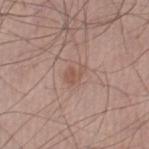{
  "image": {
    "source": "total-body photography crop",
    "field_of_view_mm": 15
  },
  "patient": {
    "sex": "male",
    "age_approx": 55
  },
  "lighting": "white-light"
}